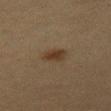biopsy_status: not biopsied; imaged during a skin examination
image:
  source: total-body photography crop
  field_of_view_mm: 15
patient:
  sex: female
  age_approx: 55
site: left thigh